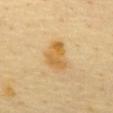{
  "biopsy_status": "not biopsied; imaged during a skin examination",
  "lesion_size": {
    "long_diameter_mm_approx": 4.0
  },
  "lighting": "cross-polarized",
  "patient": {
    "sex": "male",
    "age_approx": 65
  },
  "automated_metrics": {
    "border_irregularity_0_10": 4.0,
    "nevus_likeness_0_100": 35,
    "lesion_detection_confidence_0_100": 100
  },
  "image": {
    "source": "total-body photography crop",
    "field_of_view_mm": 15
  },
  "site": "abdomen"
}